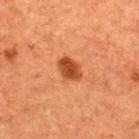| feature | finding |
|---|---|
| biopsy status | imaged on a skin check; not biopsied |
| image | ~15 mm crop, total-body skin-cancer survey |
| illumination | cross-polarized illumination |
| subject | male, roughly 50 years of age |
| anatomic site | the back |